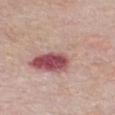The lesion was photographed on a routine skin check and not biopsied; there is no pathology result.
From the front of the torso.
A male subject aged 63–67.
A region of skin cropped from a whole-body photographic capture, roughly 15 mm wide.